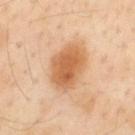<record>
<biopsy_status>not biopsied; imaged during a skin examination</biopsy_status>
<automated_metrics>
  <cielab_L>62</cielab_L>
  <cielab_a>23</cielab_a>
  <cielab_b>40</cielab_b>
  <vs_skin_darker_L>13.0</vs_skin_darker_L>
  <vs_skin_contrast_norm>8.5</vs_skin_contrast_norm>
  <nevus_likeness_0_100>90</nevus_likeness_0_100>
  <lesion_detection_confidence_0_100>100</lesion_detection_confidence_0_100>
</automated_metrics>
<image>
  <source>total-body photography crop</source>
  <field_of_view_mm>15</field_of_view_mm>
</image>
<lighting>cross-polarized</lighting>
<site>back</site>
<patient>
  <sex>male</sex>
  <age_approx>55</age_approx>
</patient>
<lesion_size>
  <long_diameter_mm_approx>5.5</long_diameter_mm_approx>
</lesion_size>
</record>